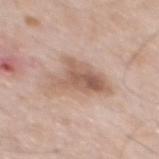Impression:
The lesion was photographed on a routine skin check and not biopsied; there is no pathology result.
Image and clinical context:
A close-up tile cropped from a whole-body skin photograph, about 15 mm across. The lesion is located on the mid back. A male subject, aged 58–62.